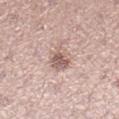Clinical impression: Part of a total-body skin-imaging series; this lesion was reviewed on a skin check and was not flagged for biopsy. Clinical summary: From the right lower leg. A 15 mm crop from a total-body photograph taken for skin-cancer surveillance. A female patient aged 33–37.